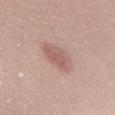biopsy status = imaged on a skin check; not biopsied
site = the chest
subject = female, in their 50s
image-analysis metrics = a lesion area of about 9.5 mm² and two-axis asymmetry of about 0.2; radial color variation of about 1
image source = ~15 mm crop, total-body skin-cancer survey
diameter = ~5 mm (longest diameter)
tile lighting = white-light